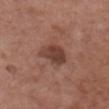Clinical impression:
Part of a total-body skin-imaging series; this lesion was reviewed on a skin check and was not flagged for biopsy.
Acquisition and patient details:
The patient is a female aged 73 to 77. Measured at roughly 4 mm in maximum diameter. This is a white-light tile. An algorithmic analysis of the crop reported a border-irregularity rating of about 2.5/10, internal color variation of about 3.5 on a 0–10 scale, and radial color variation of about 1. On the chest. A 15 mm close-up tile from a total-body photography series done for melanoma screening.